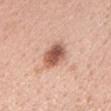The lesion is on the head or neck.
A female subject, approximately 45 years of age.
Imaged with white-light lighting.
A lesion tile, about 15 mm wide, cut from a 3D total-body photograph.